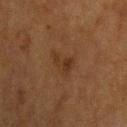Recorded during total-body skin imaging; not selected for excision or biopsy. On the upper back. This image is a 15 mm lesion crop taken from a total-body photograph. Automated tile analysis of the lesion measured a lesion area of about 5.5 mm², a shape eccentricity near 0.75, and a shape-asymmetry score of about 0.5 (0 = symmetric). It also reported a color-variation rating of about 3/10 and radial color variation of about 1.5. A male subject roughly 75 years of age. Longest diameter approximately 3.5 mm. Imaged with cross-polarized lighting.